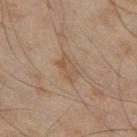| key | value |
|---|---|
| follow-up | catalogued during a skin exam; not biopsied |
| imaging modality | ~15 mm tile from a whole-body skin photo |
| subject | male, aged around 45 |
| location | the left thigh |